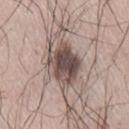The lesion was photographed on a routine skin check and not biopsied; there is no pathology result. A male patient aged 63–67. Captured under white-light illumination. The lesion is located on the lower back. An algorithmic analysis of the crop reported an area of roughly 13 mm², an outline eccentricity of about 0.6 (0 = round, 1 = elongated), and a shape-asymmetry score of about 0.2 (0 = symmetric). And it measured a nevus-likeness score of about 45/100. Measured at roughly 4.5 mm in maximum diameter. Cropped from a whole-body photographic skin survey; the tile spans about 15 mm.